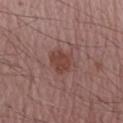follow-up = total-body-photography surveillance lesion; no biopsy | location = the right forearm | image = 15 mm crop, total-body photography | subject = male, aged 68–72.